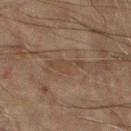{"biopsy_status": "not biopsied; imaged during a skin examination", "patient": {"sex": "male", "age_approx": 60}, "lesion_size": {"long_diameter_mm_approx": 4.0}, "image": {"source": "total-body photography crop", "field_of_view_mm": 15}, "lighting": "cross-polarized", "automated_metrics": {"shape_asymmetry": 0.4, "border_irregularity_0_10": 5.5, "color_variation_0_10": 1.0, "peripheral_color_asymmetry": 0.5}, "site": "left lower leg"}